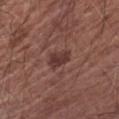{"biopsy_status": "not biopsied; imaged during a skin examination", "image": {"source": "total-body photography crop", "field_of_view_mm": 15}, "site": "left upper arm", "patient": {"sex": "male", "age_approx": 65}}